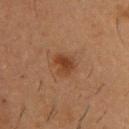Part of a total-body skin-imaging series; this lesion was reviewed on a skin check and was not flagged for biopsy.
The patient is a male in their mid-50s.
Located on the chest.
Longest diameter approximately 2.5 mm.
A 15 mm close-up tile from a total-body photography series done for melanoma screening.